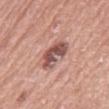Findings:
– notes · catalogued during a skin exam; not biopsied
– patient · male, roughly 75 years of age
– anatomic site · the abdomen
– image source · ~15 mm crop, total-body skin-cancer survey